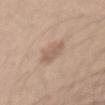Assessment: No biopsy was performed on this lesion — it was imaged during a full skin examination and was not determined to be concerning. Image and clinical context: A male subject about 30 years old. On the abdomen. A close-up tile cropped from a whole-body skin photograph, about 15 mm across. The tile uses white-light illumination.